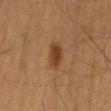| key | value |
|---|---|
| follow-up | no biopsy performed (imaged during a skin exam) |
| lesion size | about 3.5 mm |
| anatomic site | the back |
| lighting | cross-polarized |
| acquisition | ~15 mm crop, total-body skin-cancer survey |
| TBP lesion metrics | a footprint of about 5.5 mm²; a mean CIELAB color near L≈33 a*≈17 b*≈28 and a lesion–skin lightness drop of about 8; a classifier nevus-likeness of about 100/100 and a detector confidence of about 100 out of 100 that the crop contains a lesion |
| patient | male, aged around 65 |